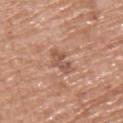Recorded during total-body skin imaging; not selected for excision or biopsy. A male subject, approximately 75 years of age. A 15 mm crop from a total-body photograph taken for skin-cancer surveillance. Located on the upper back.